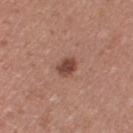biopsy status: imaged on a skin check; not biopsied
image source: ~15 mm crop, total-body skin-cancer survey
anatomic site: the arm
illumination: white-light
patient: female, approximately 40 years of age